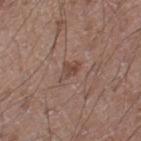<case>
  <biopsy_status>not biopsied; imaged during a skin examination</biopsy_status>
</case>